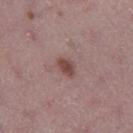Recorded during total-body skin imaging; not selected for excision or biopsy. A region of skin cropped from a whole-body photographic capture, roughly 15 mm wide. Automated image analysis of the tile measured a footprint of about 4 mm², a shape eccentricity near 0.7, and two-axis asymmetry of about 0.2. It also reported a classifier nevus-likeness of about 90/100 and a detector confidence of about 100 out of 100 that the crop contains a lesion. A female patient aged 43–47. The lesion is located on the left thigh. The lesion's longest dimension is about 2.5 mm. The tile uses white-light illumination.